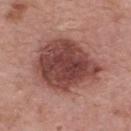  biopsy_status: not biopsied; imaged during a skin examination
  automated_metrics:
    peripheral_color_asymmetry: 1.5
  site: upper back
  image:
    source: total-body photography crop
    field_of_view_mm: 15
  patient:
    sex: male
    age_approx: 65
  lighting: white-light